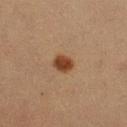No biopsy was performed on this lesion — it was imaged during a full skin examination and was not determined to be concerning.
The patient is a male about 40 years old.
This image is a 15 mm lesion crop taken from a total-body photograph.
The tile uses cross-polarized illumination.
Automated tile analysis of the lesion measured a mean CIELAB color near L≈34 a*≈19 b*≈29, about 11 CIELAB-L* units darker than the surrounding skin, and a normalized border contrast of about 10.5. It also reported a color-variation rating of about 2/10 and peripheral color asymmetry of about 0.5. The analysis additionally found a nevus-likeness score of about 100/100 and a detector confidence of about 100 out of 100 that the crop contains a lesion.
The lesion is located on the left upper arm.
Measured at roughly 2.5 mm in maximum diameter.